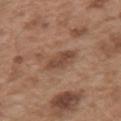| key | value |
|---|---|
| workup | total-body-photography surveillance lesion; no biopsy |
| image source | ~15 mm tile from a whole-body skin photo |
| automated metrics | border irregularity of about 2.5 on a 0–10 scale, a color-variation rating of about 2/10, and peripheral color asymmetry of about 1; a nevus-likeness score of about 10/100 and lesion-presence confidence of about 100/100 |
| body site | the left upper arm |
| diameter | ~3.5 mm (longest diameter) |
| subject | male, aged approximately 55 |